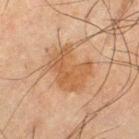biopsy_status: not biopsied; imaged during a skin examination
image:
  source: total-body photography crop
  field_of_view_mm: 15
lighting: cross-polarized
lesion_size:
  long_diameter_mm_approx: 5.0
patient:
  sex: male
  age_approx: 65
site: left thigh